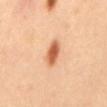<tbp_lesion>
  <biopsy_status>not biopsied; imaged during a skin examination</biopsy_status>
  <patient>
    <sex>male</sex>
    <age_approx>40</age_approx>
  </patient>
  <image>
    <source>total-body photography crop</source>
    <field_of_view_mm>15</field_of_view_mm>
  </image>
  <site>mid back</site>
  <lesion_size>
    <long_diameter_mm_approx>4.0</long_diameter_mm_approx>
  </lesion_size>
  <lighting>cross-polarized</lighting>
</tbp_lesion>